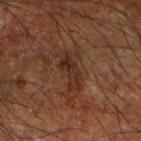Clinical impression: The lesion was tiled from a total-body skin photograph and was not biopsied. Clinical summary: A male subject, aged 63–67. Cropped from a whole-body photographic skin survey; the tile spans about 15 mm. An algorithmic analysis of the crop reported an average lesion color of about L≈24 a*≈16 b*≈22 (CIELAB), a lesion–skin lightness drop of about 6, and a lesion-to-skin contrast of about 6.5 (normalized; higher = more distinct). It also reported an automated nevus-likeness rating near 0 out of 100. From the right forearm. The recorded lesion diameter is about 5.5 mm. Imaged with cross-polarized lighting.